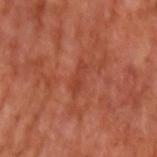| field | value |
|---|---|
| notes | no biopsy performed (imaged during a skin exam) |
| lesion size | ≈3.5 mm |
| location | the arm |
| imaging modality | ~15 mm tile from a whole-body skin photo |
| patient | male, aged approximately 55 |